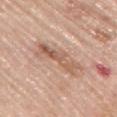Imaged during a routine full-body skin examination; the lesion was not biopsied and no histopathology is available. A 15 mm close-up extracted from a 3D total-body photography capture. A female subject, in their mid-60s. This is a white-light tile. The lesion is on the right upper arm. Longest diameter approximately 5.5 mm. An algorithmic analysis of the crop reported an outline eccentricity of about 0.9 (0 = round, 1 = elongated) and a symmetry-axis asymmetry near 0.4. The software also gave a normalized lesion–skin contrast near 7. It also reported a border-irregularity rating of about 6/10, a within-lesion color-variation index near 7.5/10, and radial color variation of about 3. The software also gave an automated nevus-likeness rating near 5 out of 100 and a detector confidence of about 100 out of 100 that the crop contains a lesion.